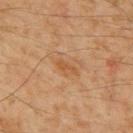Findings:
* biopsy status: imaged on a skin check; not biopsied
* patient: male, aged approximately 60
* anatomic site: the mid back
* imaging modality: total-body-photography crop, ~15 mm field of view